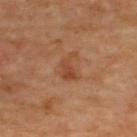No biopsy was performed on this lesion — it was imaged during a full skin examination and was not determined to be concerning. A region of skin cropped from a whole-body photographic capture, roughly 15 mm wide. The lesion is located on the upper back. Longest diameter approximately 3.5 mm.